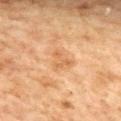biopsy_status: not biopsied; imaged during a skin examination
lesion_size:
  long_diameter_mm_approx: 2.5
lighting: cross-polarized
automated_metrics:
  area_mm2_approx: 4.0
  eccentricity: 0.45
  shape_asymmetry: 0.65
  vs_skin_darker_L: 7.0
  border_irregularity_0_10: 8.5
  color_variation_0_10: 0.0
  peripheral_color_asymmetry: 0.0
  lesion_detection_confidence_0_100: 100
image:
  source: total-body photography crop
  field_of_view_mm: 15
patient:
  sex: female
  age_approx: 60
site: back